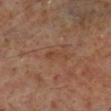The lesion was photographed on a routine skin check and not biopsied; there is no pathology result.
A roughly 15 mm field-of-view crop from a total-body skin photograph.
A male subject aged 58 to 62.
An algorithmic analysis of the crop reported border irregularity of about 5 on a 0–10 scale, a within-lesion color-variation index near 0/10, and radial color variation of about 0.
Longest diameter approximately 3 mm.
Imaged with cross-polarized lighting.
From the right lower leg.